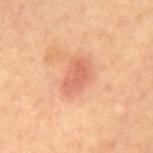biopsy_status: not biopsied; imaged during a skin examination
site: mid back
patient:
  sex: male
  age_approx: 70
image:
  source: total-body photography crop
  field_of_view_mm: 15